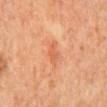Clinical impression: Captured during whole-body skin photography for melanoma surveillance; the lesion was not biopsied. Clinical summary: On the mid back. This image is a 15 mm lesion crop taken from a total-body photograph. A male patient in their mid- to late 60s.